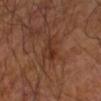notes = catalogued during a skin exam; not biopsied
illumination = cross-polarized
subject = male, in their 70s
image source = total-body-photography crop, ~15 mm field of view
site = the arm
size = ≈3 mm